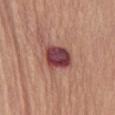The tile uses white-light illumination.
A male patient, approximately 65 years of age.
From the abdomen.
A 15 mm crop from a total-body photograph taken for skin-cancer surveillance.